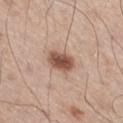Imaged during a routine full-body skin examination; the lesion was not biopsied and no histopathology is available. A 15 mm close-up tile from a total-body photography series done for melanoma screening. Longest diameter approximately 3.5 mm. Captured under white-light illumination. Located on the right thigh. A male subject aged around 60. Automated tile analysis of the lesion measured an area of roughly 7 mm² and a shape eccentricity near 0.65. The analysis additionally found an average lesion color of about L≈52 a*≈21 b*≈28 (CIELAB), roughly 16 lightness units darker than nearby skin, and a lesion-to-skin contrast of about 10.5 (normalized; higher = more distinct). The software also gave radial color variation of about 1.